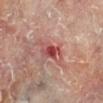The lesion was photographed on a routine skin check and not biopsied; there is no pathology result. The subject is a male roughly 60 years of age. A 15 mm crop from a total-body photograph taken for skin-cancer surveillance. Imaged with cross-polarized lighting. Longest diameter approximately 3.5 mm. On the leg.